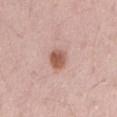Clinical impression:
Part of a total-body skin-imaging series; this lesion was reviewed on a skin check and was not flagged for biopsy.
Background:
The lesion is on the mid back. The patient is a male in their 70s. About 3 mm across. The tile uses white-light illumination. Cropped from a whole-body photographic skin survey; the tile spans about 15 mm.